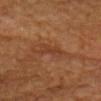No biopsy was performed on this lesion — it was imaged during a full skin examination and was not determined to be concerning.
Automated image analysis of the tile measured lesion-presence confidence of about 80/100.
Cropped from a whole-body photographic skin survey; the tile spans about 15 mm.
A male subject, in their mid- to late 80s or older.
Located on the head or neck.
The recorded lesion diameter is about 4 mm.
Captured under cross-polarized illumination.